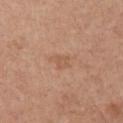workup=total-body-photography surveillance lesion; no biopsy
body site=the front of the torso
patient=male, in their mid-70s
image source=~15 mm tile from a whole-body skin photo